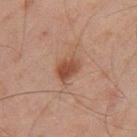No biopsy was performed on this lesion — it was imaged during a full skin examination and was not determined to be concerning.
The lesion is located on the right thigh.
A male subject in their mid-40s.
This image is a 15 mm lesion crop taken from a total-body photograph.